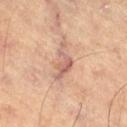This lesion was catalogued during total-body skin photography and was not selected for biopsy.
On the right thigh.
The patient is a male about 65 years old.
Longest diameter approximately 4.5 mm.
Cropped from a whole-body photographic skin survey; the tile spans about 15 mm.